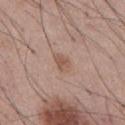Impression:
Part of a total-body skin-imaging series; this lesion was reviewed on a skin check and was not flagged for biopsy.
Context:
The lesion is located on the abdomen. A male subject, approximately 55 years of age. The lesion's longest dimension is about 2.5 mm. Automated image analysis of the tile measured a lesion area of about 3.5 mm² and two-axis asymmetry of about 0.25. The software also gave a border-irregularity rating of about 2/10, a color-variation rating of about 2/10, and radial color variation of about 1. It also reported an automated nevus-likeness rating near 40 out of 100 and a lesion-detection confidence of about 100/100. A 15 mm close-up tile from a total-body photography series done for melanoma screening. The tile uses white-light illumination.